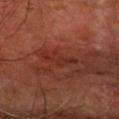Notes:
- notes — imaged on a skin check; not biopsied
- illumination — cross-polarized
- image — 15 mm crop, total-body photography
- body site — the right forearm
- automated metrics — a lesion area of about 4.5 mm² and two-axis asymmetry of about 0.55; a classifier nevus-likeness of about 0/100
- patient — aged around 65